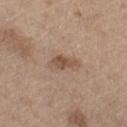<record>
  <biopsy_status>not biopsied; imaged during a skin examination</biopsy_status>
  <lighting>white-light</lighting>
  <site>left thigh</site>
  <automated_metrics>
    <area_mm2_approx>5.5</area_mm2_approx>
    <eccentricity>0.9</eccentricity>
    <shape_asymmetry>0.35</shape_asymmetry>
    <cielab_L>52</cielab_L>
    <cielab_a>17</cielab_a>
    <cielab_b>29</cielab_b>
    <vs_skin_darker_L>10.0</vs_skin_darker_L>
    <vs_skin_contrast_norm>7.0</vs_skin_contrast_norm>
    <border_irregularity_0_10>3.5</border_irregularity_0_10>
    <color_variation_0_10>3.0</color_variation_0_10>
    <peripheral_color_asymmetry>1.0</peripheral_color_asymmetry>
    <lesion_detection_confidence_0_100>100</lesion_detection_confidence_0_100>
  </automated_metrics>
  <lesion_size>
    <long_diameter_mm_approx>4.0</long_diameter_mm_approx>
  </lesion_size>
  <patient>
    <sex>male</sex>
    <age_approx>70</age_approx>
  </patient>
  <image>
    <source>total-body photography crop</source>
    <field_of_view_mm>15</field_of_view_mm>
  </image>
</record>